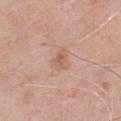| field | value |
|---|---|
| workup | catalogued during a skin exam; not biopsied |
| lesion size | ~3 mm (longest diameter) |
| site | the chest |
| patient | male, aged around 60 |
| image | total-body-photography crop, ~15 mm field of view |